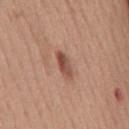{
  "biopsy_status": "not biopsied; imaged during a skin examination",
  "patient": {
    "sex": "male",
    "age_approx": 50
  },
  "site": "mid back",
  "image": {
    "source": "total-body photography crop",
    "field_of_view_mm": 15
  }
}